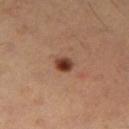biopsy_status: not biopsied; imaged during a skin examination
automated_metrics:
  eccentricity: 0.45
  shape_asymmetry: 0.15
  cielab_L: 38
  cielab_a: 22
  cielab_b: 28
  vs_skin_darker_L: 14.0
  vs_skin_contrast_norm: 11.5
  border_irregularity_0_10: 1.0
  peripheral_color_asymmetry: 1.5
site: left thigh
image:
  source: total-body photography crop
  field_of_view_mm: 15
lesion_size:
  long_diameter_mm_approx: 2.5
patient:
  sex: male
  age_approx: 55